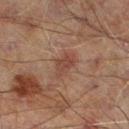Q: Was this lesion biopsied?
A: imaged on a skin check; not biopsied
Q: Where on the body is the lesion?
A: the left lower leg
Q: How was this image acquired?
A: 15 mm crop, total-body photography
Q: How was the tile lit?
A: cross-polarized illumination
Q: Lesion size?
A: about 3.5 mm
Q: Patient demographics?
A: male, approximately 60 years of age